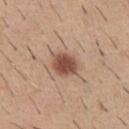Assessment:
Captured during whole-body skin photography for melanoma surveillance; the lesion was not biopsied.
Background:
Measured at roughly 3 mm in maximum diameter. A 15 mm close-up tile from a total-body photography series done for melanoma screening. The lesion is on the chest. The patient is a male aged 38 to 42. Automated image analysis of the tile measured a border-irregularity rating of about 1.5/10, a within-lesion color-variation index near 2.5/10, and a peripheral color-asymmetry measure near 0.5. The software also gave a nevus-likeness score of about 100/100 and a detector confidence of about 100 out of 100 that the crop contains a lesion.